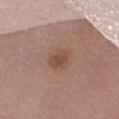Impression: The lesion was photographed on a routine skin check and not biopsied; there is no pathology result.